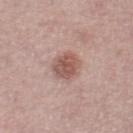notes: no biopsy performed (imaged during a skin exam) | image-analysis metrics: an area of roughly 8.5 mm², an eccentricity of roughly 0.65, and two-axis asymmetry of about 0.15; about 11 CIELAB-L* units darker than the surrounding skin and a lesion-to-skin contrast of about 7.5 (normalized; higher = more distinct) | site: the right thigh | illumination: white-light illumination | imaging modality: 15 mm crop, total-body photography | subject: female, aged around 65.